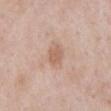workup: total-body-photography surveillance lesion; no biopsy
image: ~15 mm crop, total-body skin-cancer survey
patient: male, aged approximately 55
tile lighting: white-light
size: ~2.5 mm (longest diameter)
site: the front of the torso